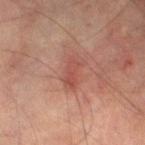No biopsy was performed on this lesion — it was imaged during a full skin examination and was not determined to be concerning. A male patient, aged around 75. On the leg. A 15 mm close-up tile from a total-body photography series done for melanoma screening. The total-body-photography lesion software estimated a lesion area of about 5.5 mm², an outline eccentricity of about 0.85 (0 = round, 1 = elongated), and a shape-asymmetry score of about 0.25 (0 = symmetric). The analysis additionally found roughly 6 lightness units darker than nearby skin and a normalized lesion–skin contrast near 5. The analysis additionally found a within-lesion color-variation index near 3/10. And it measured a classifier nevus-likeness of about 0/100 and a detector confidence of about 100 out of 100 that the crop contains a lesion. About 3.5 mm across.